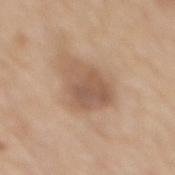Clinical impression: The lesion was photographed on a routine skin check and not biopsied; there is no pathology result. Clinical summary: Cropped from a whole-body photographic skin survey; the tile spans about 15 mm. On the mid back. The lesion-visualizer software estimated a mean CIELAB color near L≈56 a*≈18 b*≈30, about 10 CIELAB-L* units darker than the surrounding skin, and a lesion-to-skin contrast of about 7 (normalized; higher = more distinct). The analysis additionally found a border-irregularity rating of about 2.5/10 and a within-lesion color-variation index near 4/10. And it measured a classifier nevus-likeness of about 10/100 and lesion-presence confidence of about 100/100. This is a white-light tile. Longest diameter approximately 4.5 mm. A female patient aged around 65.